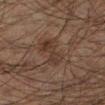follow-up = catalogued during a skin exam; not biopsied | anatomic site = the left lower leg | image source = total-body-photography crop, ~15 mm field of view | patient = male, in their 50s | TBP lesion metrics = a mean CIELAB color near L≈27 a*≈13 b*≈19, roughly 5 lightness units darker than nearby skin, and a normalized lesion–skin contrast near 5.5; border irregularity of about 4.5 on a 0–10 scale, a color-variation rating of about 3/10, and radial color variation of about 1 | lesion diameter = about 5 mm.